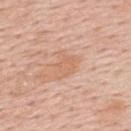A roughly 15 mm field-of-view crop from a total-body skin photograph. The subject is a male aged 53–57. The lesion is located on the back.